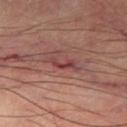Case summary:
* notes · no biopsy performed (imaged during a skin exam)
* site · the right thigh
* tile lighting · cross-polarized
* size · ~4 mm (longest diameter)
* image source · ~15 mm tile from a whole-body skin photo
* subject · male, aged approximately 65